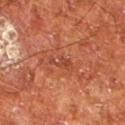Q: Was this lesion biopsied?
A: imaged on a skin check; not biopsied
Q: How was the tile lit?
A: cross-polarized illumination
Q: Patient demographics?
A: male, in their mid- to late 60s
Q: What is the lesion's diameter?
A: ≈3 mm
Q: What kind of image is this?
A: 15 mm crop, total-body photography
Q: What did automated image analysis measure?
A: a footprint of about 3.5 mm², a shape eccentricity near 0.85, and a shape-asymmetry score of about 0.5 (0 = symmetric); about 7 CIELAB-L* units darker than the surrounding skin
Q: What is the anatomic site?
A: the left lower leg